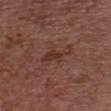Part of a total-body skin-imaging series; this lesion was reviewed on a skin check and was not flagged for biopsy.
Located on the chest.
A close-up tile cropped from a whole-body skin photograph, about 15 mm across.
This is a white-light tile.
The total-body-photography lesion software estimated a lesion area of about 6.5 mm², an eccentricity of roughly 0.9, and two-axis asymmetry of about 0.45. The software also gave a nevus-likeness score of about 0/100 and lesion-presence confidence of about 100/100.
The recorded lesion diameter is about 4.5 mm.
The patient is a female aged 63–67.